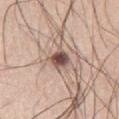The lesion was tiled from a total-body skin photograph and was not biopsied. Cropped from a total-body skin-imaging series; the visible field is about 15 mm. The lesion is on the left thigh. Imaged with white-light lighting. The patient is a male about 55 years old.